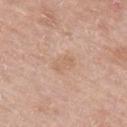The lesion was photographed on a routine skin check and not biopsied; there is no pathology result.